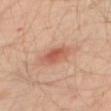| key | value |
|---|---|
| follow-up | total-body-photography surveillance lesion; no biopsy |
| subject | male, about 30 years old |
| imaging modality | ~15 mm crop, total-body skin-cancer survey |
| tile lighting | cross-polarized illumination |
| TBP lesion metrics | a lesion color around L≈55 a*≈25 b*≈30 in CIELAB, roughly 10 lightness units darker than nearby skin, and a normalized lesion–skin contrast near 7; border irregularity of about 2 on a 0–10 scale and peripheral color asymmetry of about 1; lesion-presence confidence of about 100/100 |
| size | ≈4.5 mm |
| anatomic site | the left leg |